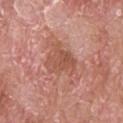No biopsy was performed on this lesion — it was imaged during a full skin examination and was not determined to be concerning. Automated image analysis of the tile measured a footprint of about 12 mm². The software also gave a lesion–skin lightness drop of about 8 and a normalized border contrast of about 6.5. The software also gave a border-irregularity rating of about 4.5/10, a within-lesion color-variation index near 3.5/10, and radial color variation of about 1.5. Cropped from a whole-body photographic skin survey; the tile spans about 15 mm. Longest diameter approximately 4.5 mm. The tile uses white-light illumination. The patient is a male in their mid-60s. From the upper back.